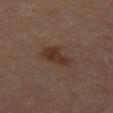Assessment:
This lesion was catalogued during total-body skin photography and was not selected for biopsy.
Background:
The recorded lesion diameter is about 4.5 mm. Located on the left thigh. Automated tile analysis of the lesion measured an area of roughly 8.5 mm², a shape eccentricity near 0.85, and a symmetry-axis asymmetry near 0.35. And it measured a mean CIELAB color near L≈31 a*≈16 b*≈23, roughly 7 lightness units darker than nearby skin, and a lesion-to-skin contrast of about 7.5 (normalized; higher = more distinct). The analysis additionally found border irregularity of about 4 on a 0–10 scale, a color-variation rating of about 2/10, and a peripheral color-asymmetry measure near 0.5. Cropped from a whole-body photographic skin survey; the tile spans about 15 mm. The tile uses cross-polarized illumination. A female subject aged 48–52.